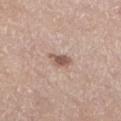Imaged during a routine full-body skin examination; the lesion was not biopsied and no histopathology is available.
A female subject, aged approximately 65.
Cropped from a total-body skin-imaging series; the visible field is about 15 mm.
From the left lower leg.
Captured under white-light illumination.
An algorithmic analysis of the crop reported a lesion area of about 3.5 mm² and an outline eccentricity of about 0.8 (0 = round, 1 = elongated). And it measured about 13 CIELAB-L* units darker than the surrounding skin and a normalized border contrast of about 8.5. The software also gave a border-irregularity rating of about 3.5/10 and internal color variation of about 1.5 on a 0–10 scale. The software also gave an automated nevus-likeness rating near 90 out of 100 and a detector confidence of about 100 out of 100 that the crop contains a lesion.